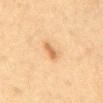This lesion was catalogued during total-body skin photography and was not selected for biopsy. Cropped from a whole-body photographic skin survey; the tile spans about 15 mm. Measured at roughly 2.5 mm in maximum diameter. The lesion-visualizer software estimated an eccentricity of roughly 0.8. And it measured a lesion color around L≈56 a*≈18 b*≈36 in CIELAB, a lesion–skin lightness drop of about 9, and a normalized lesion–skin contrast near 7. And it measured a border-irregularity rating of about 2.5/10, a color-variation rating of about 2/10, and peripheral color asymmetry of about 0.5. And it measured a nevus-likeness score of about 85/100. Located on the abdomen. A female subject, aged approximately 40.